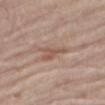Assessment:
No biopsy was performed on this lesion — it was imaged during a full skin examination and was not determined to be concerning.
Context:
A 15 mm crop from a total-body photograph taken for skin-cancer surveillance. The lesion-visualizer software estimated a lesion color around L≈54 a*≈18 b*≈27 in CIELAB, about 8 CIELAB-L* units darker than the surrounding skin, and a normalized border contrast of about 6. The software also gave a border-irregularity rating of about 5/10, a color-variation rating of about 1.5/10, and peripheral color asymmetry of about 0.5. The analysis additionally found a nevus-likeness score of about 0/100 and a lesion-detection confidence of about 85/100. The patient is a male roughly 80 years of age. Captured under white-light illumination. Longest diameter approximately 3.5 mm. Located on the right thigh.